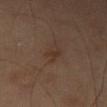Part of a total-body skin-imaging series; this lesion was reviewed on a skin check and was not flagged for biopsy. The tile uses cross-polarized illumination. Longest diameter approximately 3 mm. From the right leg. A female patient aged around 60. The lesion-visualizer software estimated an area of roughly 3.5 mm² and an outline eccentricity of about 0.85 (0 = round, 1 = elongated). The analysis additionally found a mean CIELAB color near L≈33 a*≈16 b*≈24, about 6 CIELAB-L* units darker than the surrounding skin, and a normalized lesion–skin contrast near 6. The analysis additionally found a color-variation rating of about 1.5/10 and a peripheral color-asymmetry measure near 0.5. And it measured a classifier nevus-likeness of about 5/100 and lesion-presence confidence of about 100/100. A roughly 15 mm field-of-view crop from a total-body skin photograph.